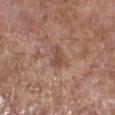Assessment:
The lesion was photographed on a routine skin check and not biopsied; there is no pathology result.